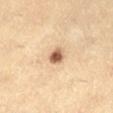Findings:
* biopsy status: imaged on a skin check; not biopsied
* image source: 15 mm crop, total-body photography
* illumination: cross-polarized illumination
* subject: female, aged approximately 40
* body site: the left lower leg
* lesion size: ~2.5 mm (longest diameter)